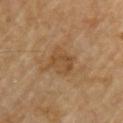Assessment:
Recorded during total-body skin imaging; not selected for excision or biopsy.
Background:
A 15 mm crop from a total-body photograph taken for skin-cancer surveillance. The tile uses cross-polarized illumination. A male patient, aged around 85 or older. Automated tile analysis of the lesion measured a lesion color around L≈46 a*≈17 b*≈35 in CIELAB and a lesion-to-skin contrast of about 5.5 (normalized; higher = more distinct). It also reported a nevus-likeness score of about 0/100 and a detector confidence of about 100 out of 100 that the crop contains a lesion. About 3.5 mm across. The lesion is located on the front of the torso.